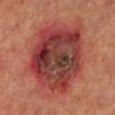Imaged during a routine full-body skin examination; the lesion was not biopsied and no histopathology is available.
The lesion's longest dimension is about 10 mm.
This image is a 15 mm lesion crop taken from a total-body photograph.
Captured under cross-polarized illumination.
From the left lower leg.
The total-body-photography lesion software estimated a mean CIELAB color near L≈42 a*≈31 b*≈27 and about 14 CIELAB-L* units darker than the surrounding skin.
A female subject, approximately 55 years of age.